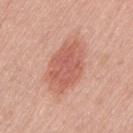Notes:
* notes: total-body-photography surveillance lesion; no biopsy
* tile lighting: white-light illumination
* size: about 7 mm
* automated lesion analysis: an area of roughly 20 mm²; border irregularity of about 2.5 on a 0–10 scale, a color-variation rating of about 3.5/10, and peripheral color asymmetry of about 1; a lesion-detection confidence of about 100/100
* patient: female, aged around 60
* location: the left thigh
* imaging modality: 15 mm crop, total-body photography